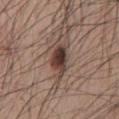Case summary:
– follow-up — imaged on a skin check; not biopsied
– patient — male, approximately 70 years of age
– image source — ~15 mm crop, total-body skin-cancer survey
– anatomic site — the mid back